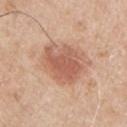Findings:
• biopsy status — no biopsy performed (imaged during a skin exam)
• acquisition — ~15 mm tile from a whole-body skin photo
• illumination — white-light illumination
• subject — male, about 65 years old
• anatomic site — the arm